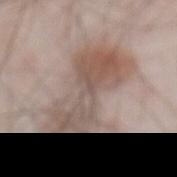The lesion was tiled from a total-body skin photograph and was not biopsied. Captured under white-light illumination. Measured at roughly 10.5 mm in maximum diameter. A male subject, aged approximately 55. From the abdomen. Automated image analysis of the tile measured a footprint of about 38 mm², a shape eccentricity near 0.85, and two-axis asymmetry of about 0.3. The software also gave border irregularity of about 5.5 on a 0–10 scale and a color-variation rating of about 7.5/10. Cropped from a total-body skin-imaging series; the visible field is about 15 mm.